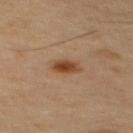follow-up: catalogued during a skin exam; not biopsied | location: the upper back | lesion diameter: ~3 mm (longest diameter) | imaging modality: total-body-photography crop, ~15 mm field of view | subject: male, aged 43–47 | tile lighting: cross-polarized illumination.